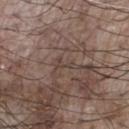Impression: Imaged during a routine full-body skin examination; the lesion was not biopsied and no histopathology is available. Acquisition and patient details: The lesion-visualizer software estimated a lesion color around L≈41 a*≈13 b*≈21 in CIELAB, roughly 5 lightness units darker than nearby skin, and a normalized lesion–skin contrast near 4.5. Cropped from a whole-body photographic skin survey; the tile spans about 15 mm. A male subject, aged around 75. From the chest.